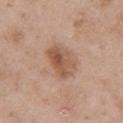The lesion was photographed on a routine skin check and not biopsied; there is no pathology result.
On the right upper arm.
Imaged with white-light lighting.
The lesion-visualizer software estimated a footprint of about 12 mm², a shape eccentricity near 0.65, and a shape-asymmetry score of about 0.2 (0 = symmetric). It also reported a within-lesion color-variation index near 6.5/10 and a peripheral color-asymmetry measure near 2.
A 15 mm close-up extracted from a 3D total-body photography capture.
The patient is a male aged 48 to 52.
Measured at roughly 4.5 mm in maximum diameter.